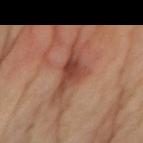Q: Was this lesion biopsied?
A: imaged on a skin check; not biopsied
Q: Who is the patient?
A: female, aged around 70
Q: How was this image acquired?
A: ~15 mm tile from a whole-body skin photo
Q: What did automated image analysis measure?
A: an automated nevus-likeness rating near 0 out of 100 and a detector confidence of about 100 out of 100 that the crop contains a lesion
Q: Illumination type?
A: cross-polarized illumination
Q: Lesion location?
A: the left forearm